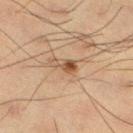{
  "biopsy_status": "not biopsied; imaged during a skin examination",
  "patient": {
    "sex": "male",
    "age_approx": 35
  },
  "site": "left lower leg",
  "image": {
    "source": "total-body photography crop",
    "field_of_view_mm": 15
  },
  "lesion_size": {
    "long_diameter_mm_approx": 2.5
  },
  "lighting": "cross-polarized"
}